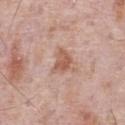Q: Is there a histopathology result?
A: catalogued during a skin exam; not biopsied
Q: What did automated image analysis measure?
A: a lesion area of about 5.5 mm², an eccentricity of roughly 0.65, and a symmetry-axis asymmetry near 0.45
Q: Where on the body is the lesion?
A: the chest
Q: What lighting was used for the tile?
A: white-light illumination
Q: Who is the patient?
A: male, in their 70s
Q: What is the lesion's diameter?
A: about 3.5 mm
Q: What kind of image is this?
A: ~15 mm tile from a whole-body skin photo